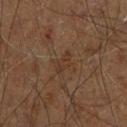notes — no biopsy performed (imaged during a skin exam)
acquisition — ~15 mm tile from a whole-body skin photo
lesion size — ≈3 mm
anatomic site — the right leg
patient — male, approximately 60 years of age